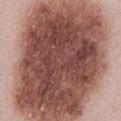Findings:
* workup · no biopsy performed (imaged during a skin exam)
* anatomic site · the abdomen
* subject · female, aged around 50
* lighting · white-light
* acquisition · 15 mm crop, total-body photography
* diameter · ~16 mm (longest diameter)